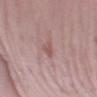Clinical impression: Captured during whole-body skin photography for melanoma surveillance; the lesion was not biopsied. Context: The lesion is located on the right forearm. Cropped from a total-body skin-imaging series; the visible field is about 15 mm. This is a white-light tile. A female patient approximately 40 years of age.